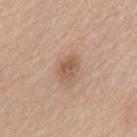Q: Was this lesion biopsied?
A: no biopsy performed (imaged during a skin exam)
Q: What is the imaging modality?
A: ~15 mm crop, total-body skin-cancer survey
Q: What is the anatomic site?
A: the back
Q: Illumination type?
A: white-light illumination
Q: Lesion size?
A: ~2.5 mm (longest diameter)
Q: What are the patient's age and sex?
A: male, roughly 65 years of age